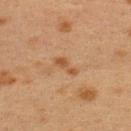{"biopsy_status": "not biopsied; imaged during a skin examination", "lighting": "cross-polarized", "patient": {"sex": "female", "age_approx": 40}, "site": "upper back", "image": {"source": "total-body photography crop", "field_of_view_mm": 15}}